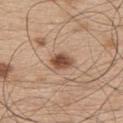- follow-up · catalogued during a skin exam; not biopsied
- anatomic site · the upper back
- subject · male, aged 48 to 52
- image · total-body-photography crop, ~15 mm field of view
- lesion size · ≈3 mm
- automated lesion analysis · a mean CIELAB color near L≈50 a*≈21 b*≈31, about 14 CIELAB-L* units darker than the surrounding skin, and a normalized border contrast of about 10; border irregularity of about 1.5 on a 0–10 scale, internal color variation of about 3.5 on a 0–10 scale, and peripheral color asymmetry of about 1.5; a nevus-likeness score of about 95/100
- tile lighting · white-light illumination